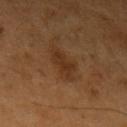Part of a total-body skin-imaging series; this lesion was reviewed on a skin check and was not flagged for biopsy. The lesion is located on the left upper arm. Cropped from a total-body skin-imaging series; the visible field is about 15 mm. A male subject aged 58 to 62.